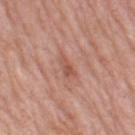TBP lesion metrics: about 8 CIELAB-L* units darker than the surrounding skin; a border-irregularity index near 3/10, internal color variation of about 0.5 on a 0–10 scale, and radial color variation of about 0; a nevus-likeness score of about 0/100 and lesion-presence confidence of about 100/100 | lesion size: about 3 mm | image source: ~15 mm crop, total-body skin-cancer survey | subject: female, aged approximately 70 | location: the left thigh | illumination: white-light illumination.